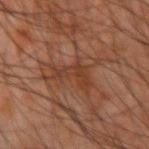Impression:
This lesion was catalogued during total-body skin photography and was not selected for biopsy.
Image and clinical context:
A roughly 15 mm field-of-view crop from a total-body skin photograph. The total-body-photography lesion software estimated an area of roughly 7.5 mm², an outline eccentricity of about 0.85 (0 = round, 1 = elongated), and two-axis asymmetry of about 0.7. The analysis additionally found a lesion color around L≈33 a*≈20 b*≈26 in CIELAB and roughly 6 lightness units darker than nearby skin. The analysis additionally found a classifier nevus-likeness of about 0/100. Imaged with cross-polarized lighting. A male patient aged 63 to 67. The lesion's longest dimension is about 4.5 mm. Located on the right forearm.